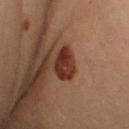Background: The recorded lesion diameter is about 4.5 mm. This is a cross-polarized tile. Cropped from a whole-body photographic skin survey; the tile spans about 15 mm. The lesion is on the left arm. A female subject, aged around 30.